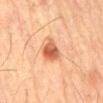Case summary:
– workup · catalogued during a skin exam; not biopsied
– patient · male, about 70 years old
– lesion diameter · ≈4 mm
– acquisition · ~15 mm crop, total-body skin-cancer survey
– tile lighting · cross-polarized
– anatomic site · the front of the torso